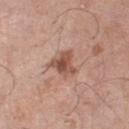The lesion was photographed on a routine skin check and not biopsied; there is no pathology result.
Imaged with white-light lighting.
Automated image analysis of the tile measured a lesion area of about 7 mm². The analysis additionally found a lesion color around L≈52 a*≈21 b*≈28 in CIELAB, a lesion–skin lightness drop of about 12, and a lesion-to-skin contrast of about 8 (normalized; higher = more distinct). The analysis additionally found a within-lesion color-variation index near 4.5/10. And it measured an automated nevus-likeness rating near 45 out of 100 and lesion-presence confidence of about 100/100.
From the left lower leg.
A male subject, approximately 70 years of age.
Cropped from a whole-body photographic skin survey; the tile spans about 15 mm.
Measured at roughly 3.5 mm in maximum diameter.